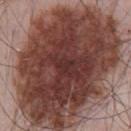Q: Is there a histopathology result?
A: no biopsy performed (imaged during a skin exam)
Q: What is the anatomic site?
A: the chest
Q: What kind of image is this?
A: total-body-photography crop, ~15 mm field of view
Q: Patient demographics?
A: male, in their mid- to late 60s